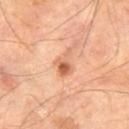<case>
  <biopsy_status>not biopsied; imaged during a skin examination</biopsy_status>
  <image>
    <source>total-body photography crop</source>
    <field_of_view_mm>15</field_of_view_mm>
  </image>
  <site>right thigh</site>
  <patient>
    <sex>male</sex>
    <age_approx>70</age_approx>
  </patient>
</case>